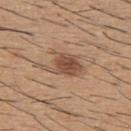The lesion was photographed on a routine skin check and not biopsied; there is no pathology result. This image is a 15 mm lesion crop taken from a total-body photograph. The subject is a male roughly 60 years of age. From the upper back.